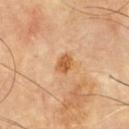The lesion was tiled from a total-body skin photograph and was not biopsied. A roughly 15 mm field-of-view crop from a total-body skin photograph. Measured at roughly 2.5 mm in maximum diameter. From the chest.